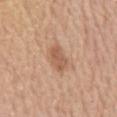biopsy status — no biopsy performed (imaged during a skin exam); automated lesion analysis — an outline eccentricity of about 0.8 (0 = round, 1 = elongated) and two-axis asymmetry of about 0.25; subject — male, aged around 80; illumination — white-light illumination; anatomic site — the mid back; lesion size — about 3 mm; image — total-body-photography crop, ~15 mm field of view.